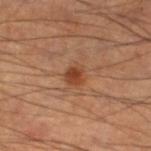Clinical impression:
This lesion was catalogued during total-body skin photography and was not selected for biopsy.
Clinical summary:
A close-up tile cropped from a whole-body skin photograph, about 15 mm across. The lesion-visualizer software estimated a border-irregularity index near 2/10, internal color variation of about 2.5 on a 0–10 scale, and peripheral color asymmetry of about 1. The recorded lesion diameter is about 2.5 mm. Imaged with cross-polarized lighting. Located on the left lower leg. A male patient, aged 58–62.